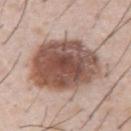No biopsy was performed on this lesion — it was imaged during a full skin examination and was not determined to be concerning.
This is a white-light tile.
Automated image analysis of the tile measured a footprint of about 42 mm², an eccentricity of roughly 0.45, and a shape-asymmetry score of about 0.15 (0 = symmetric). The software also gave a border-irregularity rating of about 2/10, a within-lesion color-variation index near 6.5/10, and radial color variation of about 2. The analysis additionally found an automated nevus-likeness rating near 90 out of 100 and a detector confidence of about 100 out of 100 that the crop contains a lesion.
The subject is a male approximately 55 years of age.
A 15 mm crop from a total-body photograph taken for skin-cancer surveillance.